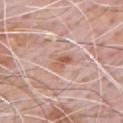follow-up = catalogued during a skin exam; not biopsied
tile lighting = white-light illumination
diameter = about 2.5 mm
subject = male, in their 80s
automated lesion analysis = a lesion area of about 4 mm², a shape eccentricity near 0.8, and a symmetry-axis asymmetry near 0.35
imaging modality = ~15 mm crop, total-body skin-cancer survey
location = the chest